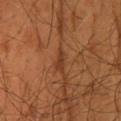notes: no biopsy performed (imaged during a skin exam).